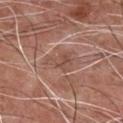biopsy status: no biopsy performed (imaged during a skin exam)
anatomic site: the chest
tile lighting: white-light illumination
subject: male, about 65 years old
image-analysis metrics: an area of roughly 5 mm², an outline eccentricity of about 0.75 (0 = round, 1 = elongated), and a shape-asymmetry score of about 0.6 (0 = symmetric); a border-irregularity rating of about 6.5/10 and a peripheral color-asymmetry measure near 1
image: ~15 mm tile from a whole-body skin photo
diameter: ≈3.5 mm